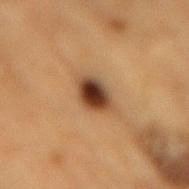notes: total-body-photography surveillance lesion; no biopsy
lighting: cross-polarized
image source: ~15 mm tile from a whole-body skin photo
patient: male, in their mid- to late 80s or older
diameter: about 4 mm
TBP lesion metrics: roughly 16 lightness units darker than nearby skin; an automated nevus-likeness rating near 100 out of 100
body site: the mid back